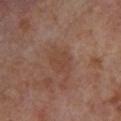Assessment: Part of a total-body skin-imaging series; this lesion was reviewed on a skin check and was not flagged for biopsy. Clinical summary: The lesion is on the right lower leg. A male subject, aged around 70. About 4 mm across. Cropped from a total-body skin-imaging series; the visible field is about 15 mm.